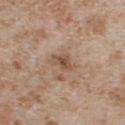Case summary:
– follow-up: no biopsy performed (imaged during a skin exam)
– subject: male, in their mid- to late 60s
– lesion size: ~3 mm (longest diameter)
– image: ~15 mm tile from a whole-body skin photo
– body site: the chest
– automated lesion analysis: a border-irregularity rating of about 4/10